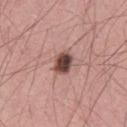Captured during whole-body skin photography for melanoma surveillance; the lesion was not biopsied. This image is a 15 mm lesion crop taken from a total-body photograph. The lesion is located on the right thigh. The lesion-visualizer software estimated a lesion area of about 6 mm², an outline eccentricity of about 0.55 (0 = round, 1 = elongated), and a symmetry-axis asymmetry near 0.25. The software also gave a nevus-likeness score of about 80/100 and lesion-presence confidence of about 100/100. Measured at roughly 3 mm in maximum diameter. This is a white-light tile. The subject is a male aged approximately 45.